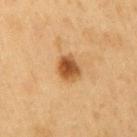biopsy status: catalogued during a skin exam; not biopsied | size: ~3 mm (longest diameter) | body site: the arm | subject: male, roughly 55 years of age | image: ~15 mm tile from a whole-body skin photo | lighting: cross-polarized.